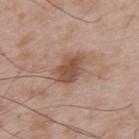Assessment: This lesion was catalogued during total-body skin photography and was not selected for biopsy. Background: Located on the mid back. A close-up tile cropped from a whole-body skin photograph, about 15 mm across. Imaged with white-light lighting. The subject is a male aged around 50. About 4 mm across. Automated tile analysis of the lesion measured a lesion area of about 8 mm² and two-axis asymmetry of about 0.25. And it measured a mean CIELAB color near L≈50 a*≈20 b*≈28, a lesion–skin lightness drop of about 11, and a normalized lesion–skin contrast near 8. It also reported border irregularity of about 3 on a 0–10 scale and internal color variation of about 2 on a 0–10 scale.